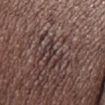Captured during whole-body skin photography for melanoma surveillance; the lesion was not biopsied.
A male subject roughly 50 years of age.
The recorded lesion diameter is about 1.5 mm.
A 15 mm crop from a total-body photograph taken for skin-cancer surveillance.
The lesion is located on the head or neck.
The lesion-visualizer software estimated a border-irregularity rating of about 3/10, a color-variation rating of about 0/10, and peripheral color asymmetry of about 0. The software also gave a classifier nevus-likeness of about 0/100 and a detector confidence of about 0 out of 100 that the crop contains a lesion.